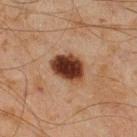{
  "biopsy_status": "not biopsied; imaged during a skin examination",
  "lesion_size": {
    "long_diameter_mm_approx": 4.5
  },
  "lighting": "cross-polarized",
  "site": "right lower leg",
  "patient": {
    "sex": "male",
    "age_approx": 45
  },
  "image": {
    "source": "total-body photography crop",
    "field_of_view_mm": 15
  }
}